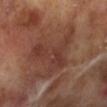| feature | finding |
|---|---|
| notes | imaged on a skin check; not biopsied |
| acquisition | 15 mm crop, total-body photography |
| patient | male, aged 68 to 72 |
| site | the left lower leg |